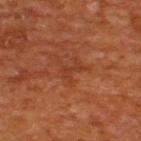Background:
Imaged with cross-polarized lighting. The lesion's longest dimension is about 2.5 mm. A 15 mm close-up tile from a total-body photography series done for melanoma screening. The lesion is on the upper back. The patient is a male about 65 years old.